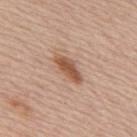Clinical impression:
The lesion was photographed on a routine skin check and not biopsied; there is no pathology result.
Background:
This is a white-light tile. A 15 mm crop from a total-body photograph taken for skin-cancer surveillance. An algorithmic analysis of the crop reported an automated nevus-likeness rating near 85 out of 100 and a detector confidence of about 100 out of 100 that the crop contains a lesion. Measured at roughly 4 mm in maximum diameter. The subject is a male approximately 80 years of age. On the upper back.